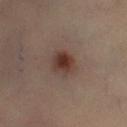Captured during whole-body skin photography for melanoma surveillance; the lesion was not biopsied.
This image is a 15 mm lesion crop taken from a total-body photograph.
From the left leg.
The patient is a female roughly 55 years of age.
About 3 mm across.
This is a cross-polarized tile.